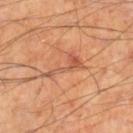biopsy status=imaged on a skin check; not biopsied
site=the right lower leg
illumination=cross-polarized illumination
patient=male, in their 70s
image source=~15 mm tile from a whole-body skin photo